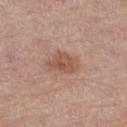{
  "biopsy_status": "not biopsied; imaged during a skin examination",
  "lesion_size": {
    "long_diameter_mm_approx": 4.0
  },
  "image": {
    "source": "total-body photography crop",
    "field_of_view_mm": 15
  },
  "patient": {
    "sex": "male",
    "age_approx": 75
  },
  "site": "leg"
}